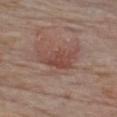Notes:
• notes — catalogued during a skin exam; not biopsied
• site — the chest
• acquisition — ~15 mm crop, total-body skin-cancer survey
• illumination — white-light illumination
• subject — male, aged 73–77